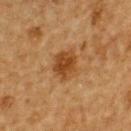Q: Is there a histopathology result?
A: total-body-photography surveillance lesion; no biopsy
Q: What are the patient's age and sex?
A: male, aged approximately 85 or older
Q: What lighting was used for the tile?
A: cross-polarized illumination
Q: What kind of image is this?
A: 15 mm crop, total-body photography
Q: What is the anatomic site?
A: the upper back